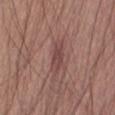biopsy status — no biopsy performed (imaged during a skin exam)
patient — male, roughly 55 years of age
acquisition — 15 mm crop, total-body photography